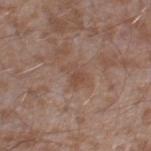• biopsy status — imaged on a skin check; not biopsied
• body site — the right thigh
• image source — 15 mm crop, total-body photography
• lighting — white-light illumination
• patient — male, aged 43 to 47